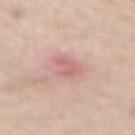workup: total-body-photography surveillance lesion; no biopsy | anatomic site: the mid back | imaging modality: ~15 mm crop, total-body skin-cancer survey | automated lesion analysis: a lesion area of about 4 mm², an outline eccentricity of about 0.8 (0 = round, 1 = elongated), and a shape-asymmetry score of about 0.3 (0 = symmetric); a border-irregularity rating of about 3.5/10 and a color-variation rating of about 1/10; a lesion-detection confidence of about 100/100 | lesion diameter: ≈3 mm | tile lighting: white-light | subject: male, in their mid- to late 50s.